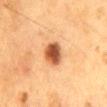{"biopsy_status": "not biopsied; imaged during a skin examination", "patient": {"sex": "male", "age_approx": 60}, "site": "back", "image": {"source": "total-body photography crop", "field_of_view_mm": 15}}